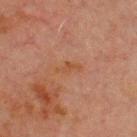Clinical summary: Located on the chest. This is a cross-polarized tile. A roughly 15 mm field-of-view crop from a total-body skin photograph. The patient is a male aged 68 to 72.